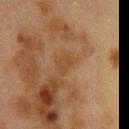biopsy status: no biopsy performed (imaged during a skin exam) | anatomic site: the chest | imaging modality: ~15 mm crop, total-body skin-cancer survey | illumination: cross-polarized | subject: female, aged 38 to 42 | diameter: ~2.5 mm (longest diameter).